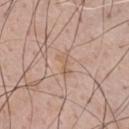<case>
<biopsy_status>not biopsied; imaged during a skin examination</biopsy_status>
<patient>
  <sex>male</sex>
  <age_approx>55</age_approx>
</patient>
<image>
  <source>total-body photography crop</source>
  <field_of_view_mm>15</field_of_view_mm>
</image>
<automated_metrics>
  <area_mm2_approx>3.5</area_mm2_approx>
  <eccentricity>0.9</eccentricity>
  <vs_skin_darker_L>6.0</vs_skin_darker_L>
  <vs_skin_contrast_norm>5.5</vs_skin_contrast_norm>
  <border_irregularity_0_10>4.5</border_irregularity_0_10>
  <color_variation_0_10>0.0</color_variation_0_10>
  <peripheral_color_asymmetry>0.0</peripheral_color_asymmetry>
  <nevus_likeness_0_100>0</nevus_likeness_0_100>
  <lesion_detection_confidence_0_100>95</lesion_detection_confidence_0_100>
</automated_metrics>
<lighting>white-light</lighting>
<site>chest</site>
</case>